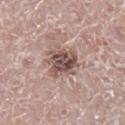The lesion was tiled from a total-body skin photograph and was not biopsied. The lesion-visualizer software estimated a footprint of about 12 mm², an outline eccentricity of about 0.6 (0 = round, 1 = elongated), and a symmetry-axis asymmetry near 0.25. And it measured a mean CIELAB color near L≈51 a*≈17 b*≈21, roughly 14 lightness units darker than nearby skin, and a normalized lesion–skin contrast near 9.5. The analysis additionally found a lesion-detection confidence of about 100/100. Located on the left leg. A male subject aged around 70. Captured under white-light illumination. A 15 mm close-up tile from a total-body photography series done for melanoma screening. The recorded lesion diameter is about 4 mm.